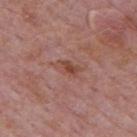workup — catalogued during a skin exam; not biopsied | image — ~15 mm crop, total-body skin-cancer survey | lighting — white-light | body site — the back | subject — male, aged around 75 | automated metrics — an area of roughly 4.5 mm², an outline eccentricity of about 0.85 (0 = round, 1 = elongated), and a symmetry-axis asymmetry near 0.35; a lesion color around L≈47 a*≈22 b*≈27 in CIELAB and a lesion-to-skin contrast of about 6.5 (normalized; higher = more distinct); a border-irregularity rating of about 4/10 and a within-lesion color-variation index near 3.5/10; lesion-presence confidence of about 100/100 | lesion diameter — ~3.5 mm (longest diameter).